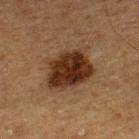{"biopsy_status": "not biopsied; imaged during a skin examination", "site": "right lower leg", "image": {"source": "total-body photography crop", "field_of_view_mm": 15}, "patient": {"sex": "male", "age_approx": 75}, "automated_metrics": {"border_irregularity_0_10": 2.5, "color_variation_0_10": 4.5}, "lighting": "cross-polarized", "lesion_size": {"long_diameter_mm_approx": 6.0}}